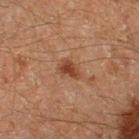Assessment:
This lesion was catalogued during total-body skin photography and was not selected for biopsy.
Image and clinical context:
Captured under cross-polarized illumination. The lesion is located on the right lower leg. About 3 mm across. A 15 mm close-up extracted from a 3D total-body photography capture. A male subject roughly 60 years of age.